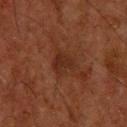Q: Was a biopsy performed?
A: imaged on a skin check; not biopsied
Q: What is the imaging modality?
A: ~15 mm tile from a whole-body skin photo
Q: What is the anatomic site?
A: the head or neck
Q: Patient demographics?
A: male, aged 63 to 67
Q: Automated lesion metrics?
A: a shape eccentricity near 0.35 and a symmetry-axis asymmetry near 0.3; an average lesion color of about L≈22 a*≈18 b*≈24 (CIELAB), a lesion–skin lightness drop of about 5, and a normalized border contrast of about 6; internal color variation of about 1.5 on a 0–10 scale and peripheral color asymmetry of about 0.5; a nevus-likeness score of about 5/100 and a detector confidence of about 100 out of 100 that the crop contains a lesion
Q: What lighting was used for the tile?
A: cross-polarized
Q: How large is the lesion?
A: ≈3 mm